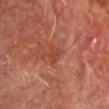Q: Automated lesion metrics?
A: an area of roughly 3 mm², an eccentricity of roughly 0.7, and two-axis asymmetry of about 0.45; an average lesion color of about L≈42 a*≈27 b*≈31 (CIELAB), roughly 6 lightness units darker than nearby skin, and a lesion-to-skin contrast of about 5.5 (normalized; higher = more distinct); a classifier nevus-likeness of about 0/100 and lesion-presence confidence of about 100/100
Q: How was this image acquired?
A: 15 mm crop, total-body photography
Q: What is the lesion's diameter?
A: ~2.5 mm (longest diameter)
Q: Illumination type?
A: cross-polarized illumination
Q: Lesion location?
A: the upper back
Q: What are the patient's age and sex?
A: male, approximately 60 years of age